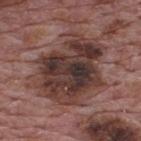Assessment:
Captured during whole-body skin photography for melanoma surveillance; the lesion was not biopsied.
Clinical summary:
This image is a 15 mm lesion crop taken from a total-body photograph. The lesion is located on the upper back. A male subject aged around 70. Approximately 9 mm at its widest. Imaged with white-light lighting.